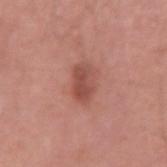| field | value |
|---|---|
| notes | imaged on a skin check; not biopsied |
| tile lighting | white-light |
| patient | male, roughly 35 years of age |
| location | the left upper arm |
| image | total-body-photography crop, ~15 mm field of view |
| image-analysis metrics | an area of roughly 7 mm², an eccentricity of roughly 0.8, and a symmetry-axis asymmetry near 0.3 |
| diameter | ≈4 mm |